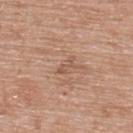<record>
  <biopsy_status>not biopsied; imaged during a skin examination</biopsy_status>
  <patient>
    <sex>female</sex>
    <age_approx>60</age_approx>
  </patient>
  <site>upper back</site>
  <image>
    <source>total-body photography crop</source>
    <field_of_view_mm>15</field_of_view_mm>
  </image>
</record>